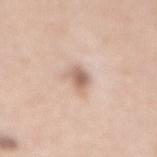| feature | finding |
|---|---|
| follow-up | total-body-photography surveillance lesion; no biopsy |
| body site | the mid back |
| lesion size | ~3 mm (longest diameter) |
| image source | total-body-photography crop, ~15 mm field of view |
| subject | female, approximately 50 years of age |